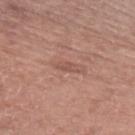biopsy_status: not biopsied; imaged during a skin examination
lighting: white-light
image:
  source: total-body photography crop
  field_of_view_mm: 15
site: arm
automated_metrics:
  cielab_L: 52
  cielab_a: 22
  cielab_b: 25
  vs_skin_darker_L: 7.0
  border_irregularity_0_10: 3.5
  color_variation_0_10: 0.0
  peripheral_color_asymmetry: 0.0
  nevus_likeness_0_100: 0
patient:
  sex: male
  age_approx: 60